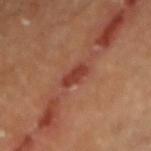| field | value |
|---|---|
| workup | imaged on a skin check; not biopsied |
| anatomic site | the right forearm |
| image | ~15 mm tile from a whole-body skin photo |
| subject | male, in their 60s |
| automated metrics | a lesion-to-skin contrast of about 7.5 (normalized; higher = more distinct); a border-irregularity index near 3/10, internal color variation of about 1.5 on a 0–10 scale, and a peripheral color-asymmetry measure near 0.5 |